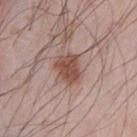Captured during whole-body skin photography for melanoma surveillance; the lesion was not biopsied.
From the chest.
A male patient, aged 63 to 67.
A lesion tile, about 15 mm wide, cut from a 3D total-body photograph.